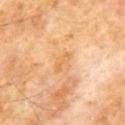The lesion was photographed on a routine skin check and not biopsied; there is no pathology result. An algorithmic analysis of the crop reported an area of roughly 3 mm², an outline eccentricity of about 0.9 (0 = round, 1 = elongated), and two-axis asymmetry of about 0.35. The analysis additionally found a mean CIELAB color near L≈65 a*≈21 b*≈43, roughly 7 lightness units darker than nearby skin, and a normalized lesion–skin contrast near 5.5. And it measured a within-lesion color-variation index near 0/10. It also reported a classifier nevus-likeness of about 0/100 and a lesion-detection confidence of about 100/100. This is a cross-polarized tile. Located on the chest. A 15 mm close-up extracted from a 3D total-body photography capture. A male patient about 60 years old. The lesion's longest dimension is about 3 mm.